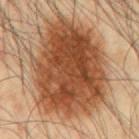{"biopsy_status": "not biopsied; imaged during a skin examination", "site": "chest", "automated_metrics": {"area_mm2_approx": 75.0, "eccentricity": 0.7, "shape_asymmetry": 0.15, "cielab_L": 46, "cielab_a": 22, "cielab_b": 34, "vs_skin_darker_L": 16.0, "border_irregularity_0_10": 3.0, "color_variation_0_10": 6.5, "peripheral_color_asymmetry": 2.5}, "patient": {"sex": "male", "age_approx": 45}, "image": {"source": "total-body photography crop", "field_of_view_mm": 15}, "lesion_size": {"long_diameter_mm_approx": 12.0}}